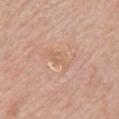Assessment:
Part of a total-body skin-imaging series; this lesion was reviewed on a skin check and was not flagged for biopsy.
Context:
A male patient, aged approximately 80. The lesion is located on the chest. About 3 mm across. Imaged with white-light lighting. Cropped from a total-body skin-imaging series; the visible field is about 15 mm.